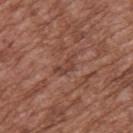image-analysis metrics: an area of roughly 3.5 mm² and a symmetry-axis asymmetry near 0.55; a border-irregularity index near 6/10 and radial color variation of about 1
site: the upper back
lesion diameter: ≈3 mm
imaging modality: total-body-photography crop, ~15 mm field of view
subject: male, approximately 75 years of age
tile lighting: white-light illumination